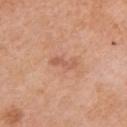A roughly 15 mm field-of-view crop from a total-body skin photograph.
Automated image analysis of the tile measured an eccentricity of roughly 0.95. The software also gave a mean CIELAB color near L≈58 a*≈25 b*≈32 and a normalized border contrast of about 5.5. The analysis additionally found border irregularity of about 5 on a 0–10 scale and internal color variation of about 0 on a 0–10 scale. The analysis additionally found a classifier nevus-likeness of about 0/100 and a lesion-detection confidence of about 100/100.
The lesion's longest dimension is about 3 mm.
From the right upper arm.
This is a white-light tile.
A female subject aged 53–57.